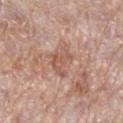follow-up: no biopsy performed (imaged during a skin exam)
diameter: ~4 mm (longest diameter)
patient: female, aged 68 to 72
automated lesion analysis: a lesion color around L≈56 a*≈21 b*≈28 in CIELAB and about 9 CIELAB-L* units darker than the surrounding skin; a classifier nevus-likeness of about 0/100 and a detector confidence of about 95 out of 100 that the crop contains a lesion
imaging modality: total-body-photography crop, ~15 mm field of view
body site: the right lower leg
lighting: white-light